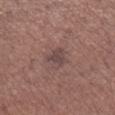<tbp_lesion>
<biopsy_status>not biopsied; imaged during a skin examination</biopsy_status>
<image>
  <source>total-body photography crop</source>
  <field_of_view_mm>15</field_of_view_mm>
</image>
<patient>
  <sex>female</sex>
  <age_approx>55</age_approx>
</patient>
<site>right lower leg</site>
</tbp_lesion>